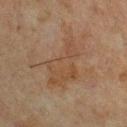The lesion was photographed on a routine skin check and not biopsied; there is no pathology result. A male subject aged approximately 65. On the upper back. A close-up tile cropped from a whole-body skin photograph, about 15 mm across. This is a cross-polarized tile.